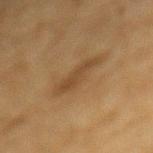workup=total-body-photography surveillance lesion; no biopsy
tile lighting=cross-polarized
imaging modality=total-body-photography crop, ~15 mm field of view
automated lesion analysis=a lesion color around L≈39 a*≈15 b*≈31 in CIELAB, a lesion–skin lightness drop of about 7, and a normalized border contrast of about 6; a border-irregularity index near 4/10, a within-lesion color-variation index near 2/10, and peripheral color asymmetry of about 0.5
size=~5 mm (longest diameter)
subject=male, aged approximately 85 or older
anatomic site=the mid back